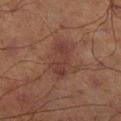Captured during whole-body skin photography for melanoma surveillance; the lesion was not biopsied. Located on the right lower leg. The subject is a male aged approximately 70. The tile uses cross-polarized illumination. Automated tile analysis of the lesion measured a lesion color around L≈29 a*≈19 b*≈20 in CIELAB, a lesion–skin lightness drop of about 6, and a normalized border contrast of about 6.5. It also reported a classifier nevus-likeness of about 20/100. Cropped from a total-body skin-imaging series; the visible field is about 15 mm.